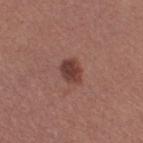notes: imaged on a skin check; not biopsied | lesion diameter: ≈3 mm | location: the right thigh | image source: 15 mm crop, total-body photography | patient: female, aged around 25.